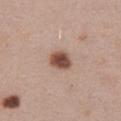Notes:
• workup: imaged on a skin check; not biopsied
• acquisition: 15 mm crop, total-body photography
• diameter: about 3 mm
• subject: female, aged 38–42
• anatomic site: the abdomen
• lighting: white-light